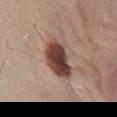Findings:
• patient: female, aged approximately 30
• site: the chest
• acquisition: ~15 mm tile from a whole-body skin photo